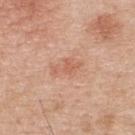  biopsy_status: not biopsied; imaged during a skin examination
  lesion_size:
    long_diameter_mm_approx: 3.5
  image:
    source: total-body photography crop
    field_of_view_mm: 15
  site: upper back
  automated_metrics:
    area_mm2_approx: 4.0
    eccentricity: 0.9
    shape_asymmetry: 0.35
  lighting: white-light
  patient:
    sex: male
    age_approx: 70